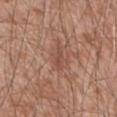workup = catalogued during a skin exam; not biopsied | lesion diameter = ~2.5 mm (longest diameter) | site = the lower back | patient = male, aged around 60 | illumination = white-light | image source = ~15 mm crop, total-body skin-cancer survey.